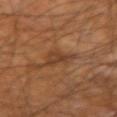{
  "biopsy_status": "not biopsied; imaged during a skin examination",
  "image": {
    "source": "total-body photography crop",
    "field_of_view_mm": 15
  },
  "lighting": "cross-polarized",
  "lesion_size": {
    "long_diameter_mm_approx": 4.5
  },
  "patient": {
    "sex": "male",
    "age_approx": 60
  },
  "site": "left forearm"
}